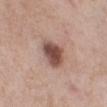A close-up tile cropped from a whole-body skin photograph, about 15 mm across. Measured at roughly 3.5 mm in maximum diameter. The patient is a female aged approximately 55. From the right lower leg. The tile uses white-light illumination.